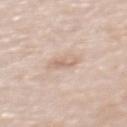Impression:
The lesion was photographed on a routine skin check and not biopsied; there is no pathology result.
Clinical summary:
A female patient about 50 years old. Captured under white-light illumination. A 15 mm crop from a total-body photograph taken for skin-cancer surveillance. Automated tile analysis of the lesion measured a shape eccentricity near 0.85. And it measured an average lesion color of about L≈67 a*≈16 b*≈28 (CIELAB), roughly 8 lightness units darker than nearby skin, and a lesion-to-skin contrast of about 5.5 (normalized; higher = more distinct). The analysis additionally found a border-irregularity rating of about 4.5/10, a within-lesion color-variation index near 1.5/10, and a peripheral color-asymmetry measure near 0.5. It also reported a classifier nevus-likeness of about 0/100 and lesion-presence confidence of about 95/100. About 3 mm across. The lesion is located on the back.